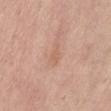The lesion was tiled from a total-body skin photograph and was not biopsied.
A lesion tile, about 15 mm wide, cut from a 3D total-body photograph.
A female subject, aged approximately 60.
On the front of the torso.